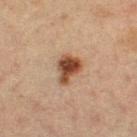follow-up = imaged on a skin check; not biopsied | lesion diameter = ≈3.5 mm | TBP lesion metrics = an average lesion color of about L≈43 a*≈20 b*≈30 (CIELAB); border irregularity of about 3.5 on a 0–10 scale and internal color variation of about 5 on a 0–10 scale | imaging modality = ~15 mm tile from a whole-body skin photo | illumination = cross-polarized | site = the left thigh | subject = female, aged around 30.